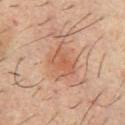Findings:
– follow-up · catalogued during a skin exam; not biopsied
– patient · male, aged approximately 35
– site · the chest
– diameter · about 4 mm
– acquisition · 15 mm crop, total-body photography